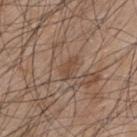Recorded during total-body skin imaging; not selected for excision or biopsy. The total-body-photography lesion software estimated an area of roughly 3.5 mm² and a symmetry-axis asymmetry near 0.3. It also reported border irregularity of about 3 on a 0–10 scale, internal color variation of about 1 on a 0–10 scale, and peripheral color asymmetry of about 0.5. It also reported a classifier nevus-likeness of about 0/100. A lesion tile, about 15 mm wide, cut from a 3D total-body photograph. Captured under white-light illumination. Longest diameter approximately 3 mm. A male patient in their mid- to late 40s. Located on the back.